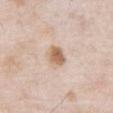Q: Illumination type?
A: white-light illumination
Q: Who is the patient?
A: male, approximately 55 years of age
Q: Where on the body is the lesion?
A: the chest
Q: How was this image acquired?
A: 15 mm crop, total-body photography